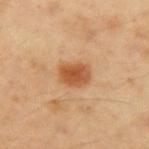Impression: The lesion was tiled from a total-body skin photograph and was not biopsied. Clinical summary: The patient is a male aged 48–52. The lesion is on the left upper arm. A region of skin cropped from a whole-body photographic capture, roughly 15 mm wide. Longest diameter approximately 3.5 mm. Imaged with cross-polarized lighting.